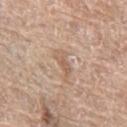Impression:
The lesion was photographed on a routine skin check and not biopsied; there is no pathology result.
Image and clinical context:
Automated tile analysis of the lesion measured an eccentricity of roughly 0.9 and a shape-asymmetry score of about 0.4 (0 = symmetric). The software also gave a lesion color around L≈60 a*≈17 b*≈29 in CIELAB and roughly 8 lightness units darker than nearby skin. And it measured border irregularity of about 4.5 on a 0–10 scale, a within-lesion color-variation index near 2.5/10, and radial color variation of about 0.5. The lesion is on the left thigh. A 15 mm close-up tile from a total-body photography series done for melanoma screening. A female patient, roughly 60 years of age. Longest diameter approximately 4 mm.